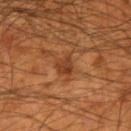Imaged during a routine full-body skin examination; the lesion was not biopsied and no histopathology is available. The lesion is located on the left forearm. Cropped from a total-body skin-imaging series; the visible field is about 15 mm. Approximately 3 mm at its widest. A male patient aged 53 to 57. The tile uses cross-polarized illumination.